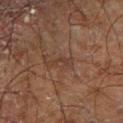This lesion was catalogued during total-body skin photography and was not selected for biopsy. The tile uses cross-polarized illumination. A region of skin cropped from a whole-body photographic capture, roughly 15 mm wide. A male patient, approximately 70 years of age. On the right forearm. Longest diameter approximately 3 mm.